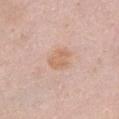workup: no biopsy performed (imaged during a skin exam) | lighting: white-light illumination | site: the front of the torso | automated lesion analysis: a border-irregularity index near 2.5/10, a color-variation rating of about 2/10, and radial color variation of about 0.5; a nevus-likeness score of about 15/100 and a lesion-detection confidence of about 100/100 | lesion diameter: ~3 mm (longest diameter) | subject: female, aged 48–52 | imaging modality: 15 mm crop, total-body photography.